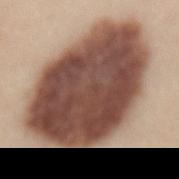{"biopsy_status": "not biopsied; imaged during a skin examination", "patient": {"sex": "female", "age_approx": 45}, "automated_metrics": {"area_mm2_approx": 70.0, "eccentricity": 0.75, "shape_asymmetry": 0.15, "cielab_L": 45, "cielab_a": 20, "cielab_b": 25, "vs_skin_darker_L": 24.0, "vs_skin_contrast_norm": 16.0, "border_irregularity_0_10": 2.0, "color_variation_0_10": 5.0, "nevus_likeness_0_100": 95, "lesion_detection_confidence_0_100": 85}, "site": "mid back", "lighting": "white-light", "image": {"source": "total-body photography crop", "field_of_view_mm": 15}, "lesion_size": {"long_diameter_mm_approx": 12.0}}